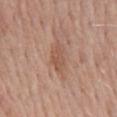This lesion was catalogued during total-body skin photography and was not selected for biopsy. The tile uses white-light illumination. On the back. Measured at roughly 3.5 mm in maximum diameter. A female patient aged around 60. A 15 mm crop from a total-body photograph taken for skin-cancer surveillance.